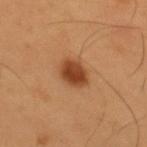biopsy_status: not biopsied; imaged during a skin examination
patient:
  sex: male
  age_approx: 55
lesion_size:
  long_diameter_mm_approx: 3.5
lighting: cross-polarized
site: upper back
image:
  source: total-body photography crop
  field_of_view_mm: 15